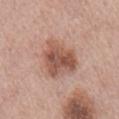Notes:
- biopsy status · total-body-photography surveillance lesion; no biopsy
- patient · male, aged around 75
- site · the back
- acquisition · ~15 mm crop, total-body skin-cancer survey
- tile lighting · white-light illumination
- image-analysis metrics · an eccentricity of roughly 0.5; a lesion color around L≈54 a*≈22 b*≈28 in CIELAB, about 12 CIELAB-L* units darker than the surrounding skin, and a normalized border contrast of about 8.5; border irregularity of about 3.5 on a 0–10 scale, a color-variation rating of about 5.5/10, and a peripheral color-asymmetry measure near 2